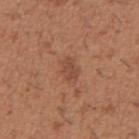Background: About 3 mm across. Captured under white-light illumination. Automated tile analysis of the lesion measured about 7 CIELAB-L* units darker than the surrounding skin and a normalized lesion–skin contrast near 5.5. The analysis additionally found a classifier nevus-likeness of about 25/100 and a detector confidence of about 100 out of 100 that the crop contains a lesion. Cropped from a whole-body photographic skin survey; the tile spans about 15 mm. A male subject, approximately 40 years of age. Located on the right upper arm.